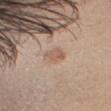Case summary:
* biopsy status · imaged on a skin check; not biopsied
* illumination · white-light illumination
* lesion diameter · ~2.5 mm (longest diameter)
* automated metrics · a footprint of about 4 mm² and a shape-asymmetry score of about 0.2 (0 = symmetric); a lesion–skin lightness drop of about 8 and a lesion-to-skin contrast of about 6 (normalized; higher = more distinct); a color-variation rating of about 1.5/10 and radial color variation of about 0.5; a nevus-likeness score of about 60/100
* patient · male, aged 33–37
* image source · total-body-photography crop, ~15 mm field of view
* anatomic site · the head or neck